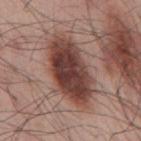Notes:
* workup · catalogued during a skin exam; not biopsied
* image source · ~15 mm tile from a whole-body skin photo
* patient · male, approximately 55 years of age
* illumination · white-light illumination
* site · the mid back